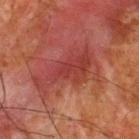This lesion was catalogued during total-body skin photography and was not selected for biopsy.
A male subject roughly 65 years of age.
Cropped from a whole-body photographic skin survey; the tile spans about 15 mm.
The lesion is on the upper back.